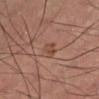This lesion was catalogued during total-body skin photography and was not selected for biopsy.
The patient is a male aged around 60.
The lesion's longest dimension is about 2.5 mm.
Automated image analysis of the tile measured border irregularity of about 2.5 on a 0–10 scale and a color-variation rating of about 2.5/10. And it measured an automated nevus-likeness rating near 15 out of 100.
This is a cross-polarized tile.
A close-up tile cropped from a whole-body skin photograph, about 15 mm across.